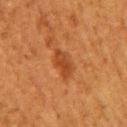subject = female, aged approximately 50 | anatomic site = the right upper arm | image-analysis metrics = a lesion area of about 5 mm², an eccentricity of roughly 0.85, and two-axis asymmetry of about 0.3; a border-irregularity index near 3/10 and a peripheral color-asymmetry measure near 0.5 | acquisition = ~15 mm crop, total-body skin-cancer survey.